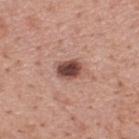The lesion was tiled from a total-body skin photograph and was not biopsied.
A region of skin cropped from a whole-body photographic capture, roughly 15 mm wide.
The subject is a male in their 40s.
Located on the upper back.